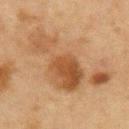Clinical summary: A 15 mm close-up tile from a total-body photography series done for melanoma screening. The lesion is on the chest. The recorded lesion diameter is about 12.5 mm. Automated image analysis of the tile measured a footprint of about 32 mm², an outline eccentricity of about 0.95 (0 = round, 1 = elongated), and a symmetry-axis asymmetry near 0.65. It also reported an average lesion color of about L≈43 a*≈17 b*≈31 (CIELAB), roughly 9 lightness units darker than nearby skin, and a lesion-to-skin contrast of about 7.5 (normalized; higher = more distinct). The software also gave a border-irregularity rating of about 9.5/10, internal color variation of about 5.5 on a 0–10 scale, and a peripheral color-asymmetry measure near 2. The analysis additionally found an automated nevus-likeness rating near 45 out of 100 and a lesion-detection confidence of about 100/100. The tile uses cross-polarized illumination. A male subject, aged 63–67.